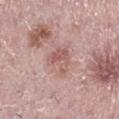Captured during whole-body skin photography for melanoma surveillance; the lesion was not biopsied.
This image is a 15 mm lesion crop taken from a total-body photograph.
Imaged with white-light lighting.
The lesion is on the leg.
A female subject roughly 40 years of age.
The lesion's longest dimension is about 3.5 mm.